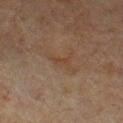No biopsy was performed on this lesion — it was imaged during a full skin examination and was not determined to be concerning.